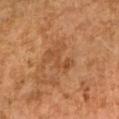workup = catalogued during a skin exam; not biopsied
location = the right upper arm
diameter = ~3.5 mm (longest diameter)
patient = female, aged around 60
lighting = cross-polarized illumination
image = 15 mm crop, total-body photography